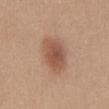| key | value |
|---|---|
| follow-up | catalogued during a skin exam; not biopsied |
| lighting | white-light |
| patient | female, in their 30s |
| anatomic site | the chest |
| acquisition | 15 mm crop, total-body photography |
| size | ≈5 mm |